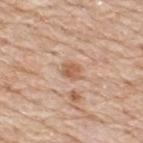Recorded during total-body skin imaging; not selected for excision or biopsy. Imaged with white-light lighting. A 15 mm close-up extracted from a 3D total-body photography capture. Longest diameter approximately 2.5 mm. Automated tile analysis of the lesion measured an eccentricity of roughly 0.6 and a symmetry-axis asymmetry near 0.3. The software also gave a classifier nevus-likeness of about 15/100 and a detector confidence of about 100 out of 100 that the crop contains a lesion. The patient is a male aged approximately 80. The lesion is on the back.